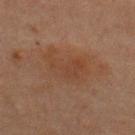Assessment:
No biopsy was performed on this lesion — it was imaged during a full skin examination and was not determined to be concerning.
Clinical summary:
The lesion is on the back. This image is a 15 mm lesion crop taken from a total-body photograph. The tile uses cross-polarized illumination. The lesion's longest dimension is about 5.5 mm. An algorithmic analysis of the crop reported an area of roughly 15 mm², a shape eccentricity near 0.8, and a shape-asymmetry score of about 0.35 (0 = symmetric). The software also gave a lesion color around L≈34 a*≈17 b*≈26 in CIELAB, about 4 CIELAB-L* units darker than the surrounding skin, and a lesion-to-skin contrast of about 5 (normalized; higher = more distinct). The subject is a male aged around 60.